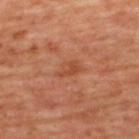location: the upper back
patient: female, in their mid- to late 40s
image-analysis metrics: an eccentricity of roughly 0.8 and two-axis asymmetry of about 0.35; an average lesion color of about L≈47 a*≈27 b*≈35 (CIELAB), roughly 7 lightness units darker than nearby skin, and a lesion-to-skin contrast of about 6 (normalized; higher = more distinct); an automated nevus-likeness rating near 0 out of 100 and a detector confidence of about 100 out of 100 that the crop contains a lesion
tile lighting: cross-polarized illumination
image source: ~15 mm tile from a whole-body skin photo